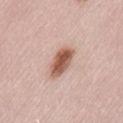Image and clinical context:
The lesion is on the lower back. The total-body-photography lesion software estimated an average lesion color of about L≈57 a*≈22 b*≈29 (CIELAB), a lesion–skin lightness drop of about 16, and a lesion-to-skin contrast of about 10.5 (normalized; higher = more distinct). And it measured a classifier nevus-likeness of about 100/100. A female subject aged approximately 70. This image is a 15 mm lesion crop taken from a total-body photograph. Captured under white-light illumination. Measured at roughly 4.5 mm in maximum diameter.